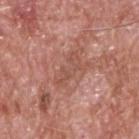The lesion was tiled from a total-body skin photograph and was not biopsied.
A male patient, aged 58 to 62.
Automated tile analysis of the lesion measured an automated nevus-likeness rating near 0 out of 100 and lesion-presence confidence of about 90/100.
The tile uses white-light illumination.
A 15 mm close-up tile from a total-body photography series done for melanoma screening.
On the upper back.
Longest diameter approximately 4 mm.